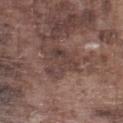notes = total-body-photography surveillance lesion; no biopsy | automated metrics = a footprint of about 9 mm², a shape eccentricity near 0.55, and a symmetry-axis asymmetry near 0.5; border irregularity of about 6 on a 0–10 scale, a color-variation rating of about 5.5/10, and a peripheral color-asymmetry measure near 2 | site = the left lower leg | patient = male, aged 73–77 | image = ~15 mm tile from a whole-body skin photo.